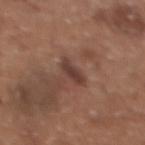The lesion was photographed on a routine skin check and not biopsied; there is no pathology result.
Captured under white-light illumination.
A female subject about 35 years old.
A lesion tile, about 15 mm wide, cut from a 3D total-body photograph.
The lesion's longest dimension is about 3 mm.
On the chest.